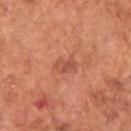The lesion is on the upper back. The lesion-visualizer software estimated roughly 8 lightness units darker than nearby skin. The software also gave border irregularity of about 3 on a 0–10 scale. The software also gave an automated nevus-likeness rating near 20 out of 100 and a lesion-detection confidence of about 100/100. The recorded lesion diameter is about 2.5 mm. A roughly 15 mm field-of-view crop from a total-body skin photograph. The patient is a male in their mid-60s.